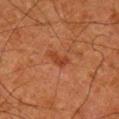follow-up — catalogued during a skin exam; not biopsied | lighting — cross-polarized | diameter — about 2.5 mm | patient — male, aged 78–82 | image — ~15 mm tile from a whole-body skin photo | body site — the right lower leg | automated lesion analysis — an area of roughly 3.5 mm² and two-axis asymmetry of about 0.45; a lesion color around L≈33 a*≈24 b*≈31 in CIELAB, roughly 7 lightness units darker than nearby skin, and a normalized lesion–skin contrast near 6.5; a nevus-likeness score of about 5/100 and a detector confidence of about 100 out of 100 that the crop contains a lesion.